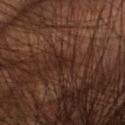notes = imaged on a skin check; not biopsied | automated lesion analysis = an average lesion color of about L≈23 a*≈17 b*≈22 (CIELAB), roughly 6 lightness units darker than nearby skin, and a normalized border contrast of about 7; border irregularity of about 5 on a 0–10 scale, a within-lesion color-variation index near 1.5/10, and radial color variation of about 0.5; an automated nevus-likeness rating near 0 out of 100 and a lesion-detection confidence of about 60/100 | diameter = ≈2.5 mm | anatomic site = the arm | subject = male, roughly 60 years of age | acquisition = ~15 mm tile from a whole-body skin photo | tile lighting = cross-polarized.